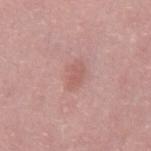Captured during whole-body skin photography for melanoma surveillance; the lesion was not biopsied.
Measured at roughly 3 mm in maximum diameter.
The subject is a male aged 48 to 52.
A 15 mm close-up tile from a total-body photography series done for melanoma screening.
The lesion-visualizer software estimated a mean CIELAB color near L≈58 a*≈23 b*≈24, roughly 7 lightness units darker than nearby skin, and a normalized border contrast of about 5. It also reported a border-irregularity rating of about 2.5/10, a within-lesion color-variation index near 1.5/10, and a peripheral color-asymmetry measure near 0.5. It also reported a detector confidence of about 100 out of 100 that the crop contains a lesion.
The lesion is on the back.